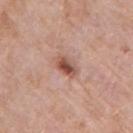notes: no biopsy performed (imaged during a skin exam) | location: the right upper arm | image: total-body-photography crop, ~15 mm field of view | patient: female, aged around 70 | lighting: white-light | diameter: ≈3 mm.